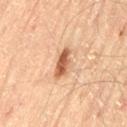<record>
<biopsy_status>not biopsied; imaged during a skin examination</biopsy_status>
<lesion_size>
  <long_diameter_mm_approx>4.0</long_diameter_mm_approx>
</lesion_size>
<patient>
  <sex>male</sex>
  <age_approx>65</age_approx>
</patient>
<image>
  <source>total-body photography crop</source>
  <field_of_view_mm>15</field_of_view_mm>
</image>
<lighting>cross-polarized</lighting>
<site>leg</site>
</record>